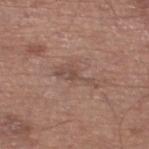Q: Is there a histopathology result?
A: catalogued during a skin exam; not biopsied
Q: Lesion location?
A: the left lower leg
Q: Illumination type?
A: white-light illumination
Q: How was this image acquired?
A: ~15 mm crop, total-body skin-cancer survey
Q: Who is the patient?
A: male, aged 63–67